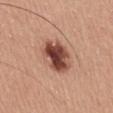biopsy status = total-body-photography surveillance lesion; no biopsy.